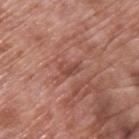Notes:
• biopsy status · total-body-photography surveillance lesion; no biopsy
• lesion size · about 2.5 mm
• location · the upper back
• subject · male, about 70 years old
• automated lesion analysis · a lesion area of about 3 mm² and a symmetry-axis asymmetry near 0.35; an average lesion color of about L≈47 a*≈25 b*≈27 (CIELAB) and a lesion-to-skin contrast of about 6 (normalized; higher = more distinct); a border-irregularity rating of about 4/10 and peripheral color asymmetry of about 0; an automated nevus-likeness rating near 0 out of 100 and a lesion-detection confidence of about 90/100
• image source · ~15 mm tile from a whole-body skin photo
• tile lighting · white-light